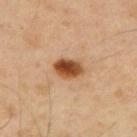The lesion was photographed on a routine skin check and not biopsied; there is no pathology result. Located on the mid back. Automated tile analysis of the lesion measured a footprint of about 6.5 mm², an eccentricity of roughly 0.75, and a symmetry-axis asymmetry near 0.15. And it measured a nevus-likeness score of about 100/100 and a lesion-detection confidence of about 100/100. A male subject approximately 40 years of age. A roughly 15 mm field-of-view crop from a total-body skin photograph. Imaged with cross-polarized lighting.